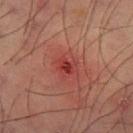Case summary:
- biopsy status · imaged on a skin check; not biopsied
- acquisition · ~15 mm crop, total-body skin-cancer survey
- subject · male, roughly 60 years of age
- diameter · about 2.5 mm
- automated metrics · an area of roughly 4 mm², an eccentricity of roughly 0.6, and two-axis asymmetry of about 0.3; a within-lesion color-variation index near 3.5/10
- tile lighting · cross-polarized